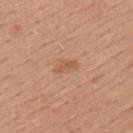Q: Was a biopsy performed?
A: total-body-photography surveillance lesion; no biopsy
Q: What kind of image is this?
A: 15 mm crop, total-body photography
Q: What are the patient's age and sex?
A: male, aged 53 to 57
Q: What is the anatomic site?
A: the upper back
Q: How large is the lesion?
A: ~3 mm (longest diameter)
Q: Automated lesion metrics?
A: an average lesion color of about L≈56 a*≈23 b*≈33 (CIELAB), about 8 CIELAB-L* units darker than the surrounding skin, and a normalized border contrast of about 5.5; a border-irregularity index near 3.5/10, a within-lesion color-variation index near 1.5/10, and a peripheral color-asymmetry measure near 0.5; an automated nevus-likeness rating near 0 out of 100 and a lesion-detection confidence of about 100/100